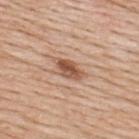Part of a total-body skin-imaging series; this lesion was reviewed on a skin check and was not flagged for biopsy.
From the upper back.
A male subject aged 58–62.
Cropped from a total-body skin-imaging series; the visible field is about 15 mm.